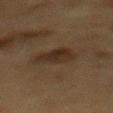The lesion was tiled from a total-body skin photograph and was not biopsied. This is a cross-polarized tile. The recorded lesion diameter is about 4.5 mm. A male patient in their mid-80s or older. Located on the mid back. The lesion-visualizer software estimated a within-lesion color-variation index near 3/10 and radial color variation of about 1. The software also gave a nevus-likeness score of about 55/100 and a detector confidence of about 100 out of 100 that the crop contains a lesion. A 15 mm crop from a total-body photograph taken for skin-cancer surveillance.